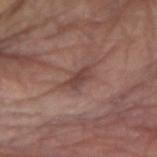Clinical impression: Part of a total-body skin-imaging series; this lesion was reviewed on a skin check and was not flagged for biopsy. Background: A region of skin cropped from a whole-body photographic capture, roughly 15 mm wide. The lesion-visualizer software estimated a footprint of about 4.5 mm² and an eccentricity of roughly 0.85. The software also gave a border-irregularity index near 4.5/10, a within-lesion color-variation index near 2/10, and peripheral color asymmetry of about 0.5. The software also gave an automated nevus-likeness rating near 0 out of 100 and a detector confidence of about 85 out of 100 that the crop contains a lesion. A male patient, aged around 80. From the right upper arm. Captured under white-light illumination.